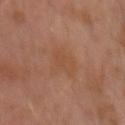Clinical impression: Recorded during total-body skin imaging; not selected for excision or biopsy. Image and clinical context: From the left upper arm. A lesion tile, about 15 mm wide, cut from a 3D total-body photograph. A male patient, about 30 years old.